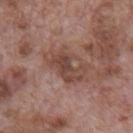Q: Was a biopsy performed?
A: imaged on a skin check; not biopsied
Q: Illumination type?
A: white-light illumination
Q: What is the anatomic site?
A: the mid back
Q: What kind of image is this?
A: ~15 mm crop, total-body skin-cancer survey
Q: What are the patient's age and sex?
A: male, aged 68 to 72